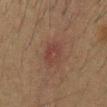notes: no biopsy performed (imaged during a skin exam)
acquisition: ~15 mm tile from a whole-body skin photo
TBP lesion metrics: a footprint of about 7 mm², a shape eccentricity near 0.75, and two-axis asymmetry of about 0.2; border irregularity of about 2.5 on a 0–10 scale and internal color variation of about 2.5 on a 0–10 scale; a nevus-likeness score of about 35/100 and a detector confidence of about 100 out of 100 that the crop contains a lesion
lighting: cross-polarized
patient: male, in their mid- to late 30s
site: the abdomen